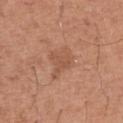Q: Is there a histopathology result?
A: no biopsy performed (imaged during a skin exam)
Q: Lesion location?
A: the left thigh
Q: What did automated image analysis measure?
A: a mean CIELAB color near L≈53 a*≈23 b*≈32 and a normalized border contrast of about 5; a classifier nevus-likeness of about 0/100 and lesion-presence confidence of about 100/100
Q: Who is the patient?
A: male, in their mid-60s
Q: What kind of image is this?
A: 15 mm crop, total-body photography